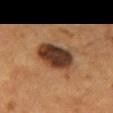Assessment:
The lesion was tiled from a total-body skin photograph and was not biopsied.
Background:
Measured at roughly 6 mm in maximum diameter. A male patient in their mid-50s. A 15 mm close-up tile from a total-body photography series done for melanoma screening. The lesion is located on the mid back.